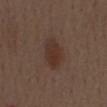Clinical impression:
Recorded during total-body skin imaging; not selected for excision or biopsy.
Context:
Imaged with white-light lighting. A male patient aged 68–72. Measured at roughly 4.5 mm in maximum diameter. An algorithmic analysis of the crop reported a lesion area of about 8.5 mm², an outline eccentricity of about 0.85 (0 = round, 1 = elongated), and a symmetry-axis asymmetry near 0.25. The software also gave roughly 7 lightness units darker than nearby skin and a normalized border contrast of about 7.5. The analysis additionally found a detector confidence of about 100 out of 100 that the crop contains a lesion. On the mid back. A roughly 15 mm field-of-view crop from a total-body skin photograph.